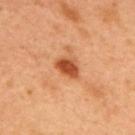| feature | finding |
|---|---|
| workup | no biopsy performed (imaged during a skin exam) |